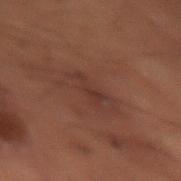{"biopsy_status": "not biopsied; imaged during a skin examination", "site": "right thigh", "lesion_size": {"long_diameter_mm_approx": 3.5}, "patient": {"sex": "male", "age_approx": 50}, "image": {"source": "total-body photography crop", "field_of_view_mm": 15}}